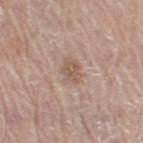Notes:
- workup · no biopsy performed (imaged during a skin exam)
- diameter · ≈2.5 mm
- subject · male, aged 68 to 72
- automated lesion analysis · a footprint of about 4 mm² and a shape eccentricity near 0.7; a lesion color around L≈55 a*≈17 b*≈27 in CIELAB and roughly 9 lightness units darker than nearby skin; a border-irregularity rating of about 2.5/10; a nevus-likeness score of about 5/100 and a detector confidence of about 100 out of 100 that the crop contains a lesion
- site · the left leg
- imaging modality · 15 mm crop, total-body photography